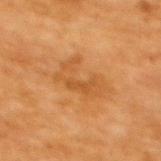biopsy status: no biopsy performed (imaged during a skin exam) | size: ≈5.5 mm | imaging modality: 15 mm crop, total-body photography | automated metrics: a lesion color around L≈46 a*≈22 b*≈39 in CIELAB and roughly 7 lightness units darker than nearby skin; border irregularity of about 7.5 on a 0–10 scale and radial color variation of about 0.5 | lighting: cross-polarized illumination | patient: female, roughly 55 years of age | site: the upper back.